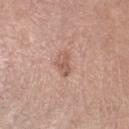Captured during whole-body skin photography for melanoma surveillance; the lesion was not biopsied. Automated image analysis of the tile measured an eccentricity of roughly 0.85 and two-axis asymmetry of about 0.35. And it measured an average lesion color of about L≈57 a*≈21 b*≈27 (CIELAB) and about 9 CIELAB-L* units darker than the surrounding skin. The patient is a female aged 68 to 72. A 15 mm crop from a total-body photograph taken for skin-cancer surveillance. The recorded lesion diameter is about 3 mm. The lesion is located on the left lower leg.